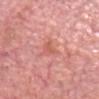Q: Was this lesion biopsied?
A: no biopsy performed (imaged during a skin exam)
Q: What are the patient's age and sex?
A: male, aged 78–82
Q: What lighting was used for the tile?
A: white-light illumination
Q: What is the imaging modality?
A: 15 mm crop, total-body photography
Q: Lesion location?
A: the head or neck
Q: Lesion size?
A: ~2.5 mm (longest diameter)